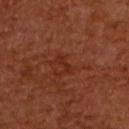Impression: The lesion was tiled from a total-body skin photograph and was not biopsied. Context: Imaged with cross-polarized lighting. A male subject, approximately 65 years of age. From the upper back. The recorded lesion diameter is about 2.5 mm. This image is a 15 mm lesion crop taken from a total-body photograph.